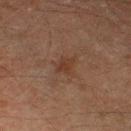Part of a total-body skin-imaging series; this lesion was reviewed on a skin check and was not flagged for biopsy.
A male patient, aged approximately 60.
The lesion is located on the left thigh.
The lesion-visualizer software estimated an area of roughly 4.5 mm², an outline eccentricity of about 0.7 (0 = round, 1 = elongated), and a symmetry-axis asymmetry near 0.35. It also reported a border-irregularity index near 4/10. It also reported an automated nevus-likeness rating near 5 out of 100 and a detector confidence of about 100 out of 100 that the crop contains a lesion.
Longest diameter approximately 3 mm.
This image is a 15 mm lesion crop taken from a total-body photograph.